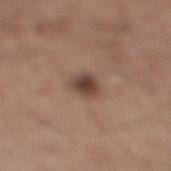Recorded during total-body skin imaging; not selected for excision or biopsy. The lesion is located on the right lower leg. A 15 mm crop from a total-body photograph taken for skin-cancer surveillance. The tile uses white-light illumination. About 3 mm across. A male subject aged around 65.